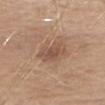Case summary:
• follow-up: no biopsy performed (imaged during a skin exam)
• image source: 15 mm crop, total-body photography
• TBP lesion metrics: a lesion area of about 7.5 mm² and a shape eccentricity near 0.75; about 9 CIELAB-L* units darker than the surrounding skin
• anatomic site: the head or neck
• lesion diameter: ≈4 mm
• subject: female, in their 40s